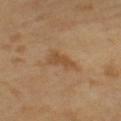Assessment: The lesion was photographed on a routine skin check and not biopsied; there is no pathology result. Acquisition and patient details: Automated image analysis of the tile measured a footprint of about 5.5 mm², an outline eccentricity of about 0.9 (0 = round, 1 = elongated), and a shape-asymmetry score of about 0.35 (0 = symmetric). It also reported a normalized border contrast of about 6. And it measured border irregularity of about 4 on a 0–10 scale, a color-variation rating of about 1.5/10, and radial color variation of about 0.5. The software also gave an automated nevus-likeness rating near 0 out of 100 and a detector confidence of about 100 out of 100 that the crop contains a lesion. The subject is a male roughly 65 years of age. This is a cross-polarized tile. Longest diameter approximately 4 mm. A 15 mm crop from a total-body photograph taken for skin-cancer surveillance.